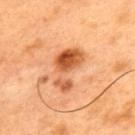This lesion was catalogued during total-body skin photography and was not selected for biopsy.
Cropped from a whole-body photographic skin survey; the tile spans about 15 mm.
The lesion-visualizer software estimated a shape-asymmetry score of about 0.5 (0 = symmetric). The analysis additionally found a lesion color around L≈58 a*≈30 b*≈43 in CIELAB, about 15 CIELAB-L* units darker than the surrounding skin, and a normalized border contrast of about 9.
About 5 mm across.
The lesion is on the upper back.
The tile uses cross-polarized illumination.
A male subject approximately 50 years of age.